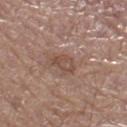{"biopsy_status": "not biopsied; imaged during a skin examination", "patient": {"sex": "male", "age_approx": 70}, "site": "right lower leg", "lesion_size": {"long_diameter_mm_approx": 3.0}, "lighting": "white-light", "automated_metrics": {"area_mm2_approx": 5.5, "eccentricity": 0.65, "shape_asymmetry": 0.35, "cielab_L": 49, "cielab_a": 18, "cielab_b": 25, "vs_skin_darker_L": 7.0, "vs_skin_contrast_norm": 5.5}, "image": {"source": "total-body photography crop", "field_of_view_mm": 15}}